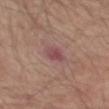<case>
<biopsy_status>not biopsied; imaged during a skin examination</biopsy_status>
<lighting>white-light</lighting>
<image>
  <source>total-body photography crop</source>
  <field_of_view_mm>15</field_of_view_mm>
</image>
<site>left forearm</site>
<lesion_size>
  <long_diameter_mm_approx>3.0</long_diameter_mm_approx>
</lesion_size>
<patient>
  <sex>male</sex>
  <age_approx>65</age_approx>
</patient>
</case>